A roughly 15 mm field-of-view crop from a total-body skin photograph. A female subject aged 53 to 57. Measured at roughly 3 mm in maximum diameter. This is a cross-polarized tile. The lesion is on the left lower leg — 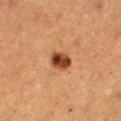Histopathological examination showed a dysplastic (Clark) nevus.